A region of skin cropped from a whole-body photographic capture, roughly 15 mm wide · the lesion is on the front of the torso · a male patient, in their mid-60s.
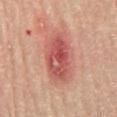automated lesion analysis: a footprint of about 17 mm² and a shape-asymmetry score of about 0.15 (0 = symmetric); a lesion color around L≈53 a*≈29 b*≈26 in CIELAB and a normalized lesion–skin contrast near 8; border irregularity of about 2 on a 0–10 scale, a color-variation rating of about 7/10, and radial color variation of about 2.5; a classifier nevus-likeness of about 55/100 and lesion-presence confidence of about 100/100
lighting: cross-polarized illumination
histopathology: a nodular basal cell carcinoma — a malignant skin lesion